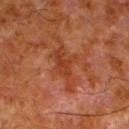Impression: The lesion was photographed on a routine skin check and not biopsied; there is no pathology result. Clinical summary: A male subject aged around 80. The tile uses cross-polarized illumination. Cropped from a whole-body photographic skin survey; the tile spans about 15 mm. On the left lower leg. The total-body-photography lesion software estimated a lesion area of about 8.5 mm², an outline eccentricity of about 0.85 (0 = round, 1 = elongated), and a shape-asymmetry score of about 0.45 (0 = symmetric). And it measured an average lesion color of about L≈31 a*≈24 b*≈29 (CIELAB). It also reported an automated nevus-likeness rating near 0 out of 100 and lesion-presence confidence of about 100/100.